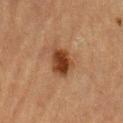Context:
The lesion is located on the abdomen. The lesion's longest dimension is about 4 mm. Cropped from a total-body skin-imaging series; the visible field is about 15 mm. A male patient roughly 85 years of age or older. The tile uses cross-polarized illumination.